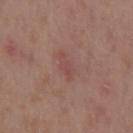Impression: Imaged during a routine full-body skin examination; the lesion was not biopsied and no histopathology is available. Background: This is a white-light tile. Longest diameter approximately 3 mm. From the mid back. The subject is a male roughly 55 years of age. A close-up tile cropped from a whole-body skin photograph, about 15 mm across.